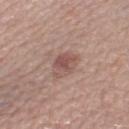notes: total-body-photography surveillance lesion; no biopsy
body site: the left lower leg
size: ~3.5 mm (longest diameter)
image source: total-body-photography crop, ~15 mm field of view
patient: female, in their mid-60s
lighting: white-light illumination